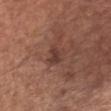Captured during whole-body skin photography for melanoma surveillance; the lesion was not biopsied.
A male subject, aged 63 to 67.
The recorded lesion diameter is about 3 mm.
A 15 mm crop from a total-body photograph taken for skin-cancer surveillance.
The lesion is located on the chest.
The lesion-visualizer software estimated internal color variation of about 1.5 on a 0–10 scale.
The tile uses white-light illumination.